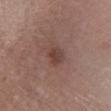– biopsy status: no biopsy performed (imaged during a skin exam)
– location: the right lower leg
– image: total-body-photography crop, ~15 mm field of view
– patient: male, approximately 55 years of age
– tile lighting: white-light illumination
– image-analysis metrics: an area of roughly 4.5 mm², an eccentricity of roughly 0.65, and two-axis asymmetry of about 0.2; an average lesion color of about L≈41 a*≈20 b*≈23 (CIELAB), a lesion–skin lightness drop of about 8, and a normalized border contrast of about 7; border irregularity of about 2 on a 0–10 scale and peripheral color asymmetry of about 1; lesion-presence confidence of about 100/100
– diameter: about 2.5 mm